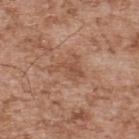Q: Was this lesion biopsied?
A: catalogued during a skin exam; not biopsied
Q: What lighting was used for the tile?
A: white-light
Q: Patient demographics?
A: male, in their mid-50s
Q: Automated lesion metrics?
A: a mean CIELAB color near L≈51 a*≈22 b*≈31, about 7 CIELAB-L* units darker than the surrounding skin, and a normalized border contrast of about 5.5; a border-irregularity rating of about 8/10, a color-variation rating of about 2/10, and peripheral color asymmetry of about 1; lesion-presence confidence of about 100/100
Q: Where on the body is the lesion?
A: the back
Q: What is the imaging modality?
A: ~15 mm tile from a whole-body skin photo
Q: How large is the lesion?
A: ≈3.5 mm